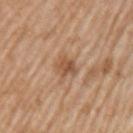Impression: Captured during whole-body skin photography for melanoma surveillance; the lesion was not biopsied. Image and clinical context: A male patient, in their 60s. About 2.5 mm across. Located on the left upper arm. A close-up tile cropped from a whole-body skin photograph, about 15 mm across.